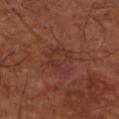| key | value |
|---|---|
| biopsy status | total-body-photography surveillance lesion; no biopsy |
| lighting | cross-polarized illumination |
| site | the right lower leg |
| acquisition | total-body-photography crop, ~15 mm field of view |
| diameter | about 4 mm |
| automated metrics | an average lesion color of about L≈33 a*≈22 b*≈26 (CIELAB), roughly 5 lightness units darker than nearby skin, and a normalized border contrast of about 5; a classifier nevus-likeness of about 0/100 and a detector confidence of about 100 out of 100 that the crop contains a lesion |
| subject | male, aged approximately 60 |